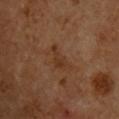Background: A 15 mm close-up extracted from a 3D total-body photography capture. The lesion is located on the upper back. A male subject, aged 58 to 62.